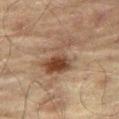{"biopsy_status": "not biopsied; imaged during a skin examination", "site": "right thigh", "image": {"source": "total-body photography crop", "field_of_view_mm": 15}, "lighting": "cross-polarized", "patient": {"sex": "male", "age_approx": 70}}